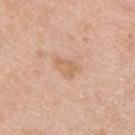Captured during whole-body skin photography for melanoma surveillance; the lesion was not biopsied. Measured at roughly 3.5 mm in maximum diameter. A 15 mm close-up tile from a total-body photography series done for melanoma screening. A male subject aged approximately 35. The tile uses white-light illumination. The lesion is on the back. The lesion-visualizer software estimated an automated nevus-likeness rating near 5 out of 100 and lesion-presence confidence of about 100/100.